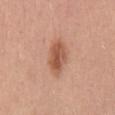* notes · imaged on a skin check; not biopsied
* subject · male, about 50 years old
* anatomic site · the mid back
* image-analysis metrics · an average lesion color of about L≈55 a*≈24 b*≈32 (CIELAB), a lesion–skin lightness drop of about 12, and a lesion-to-skin contrast of about 8 (normalized; higher = more distinct); a border-irregularity rating of about 2/10, a color-variation rating of about 5/10, and peripheral color asymmetry of about 1.5; a classifier nevus-likeness of about 95/100 and a lesion-detection confidence of about 100/100
* imaging modality · ~15 mm tile from a whole-body skin photo
* lighting · white-light
* lesion size · ~4.5 mm (longest diameter)